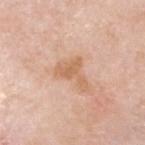No biopsy was performed on this lesion — it was imaged during a full skin examination and was not determined to be concerning. The subject is a male aged around 80. Cropped from a total-body skin-imaging series; the visible field is about 15 mm. About 4 mm across. The lesion is located on the head or neck.